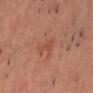{
  "biopsy_status": "not biopsied; imaged during a skin examination",
  "lesion_size": {
    "long_diameter_mm_approx": 3.0
  },
  "image": {
    "source": "total-body photography crop",
    "field_of_view_mm": 15
  },
  "automated_metrics": {
    "area_mm2_approx": 3.0,
    "shape_asymmetry": 0.35,
    "nevus_likeness_0_100": 0,
    "lesion_detection_confidence_0_100": 100
  },
  "lighting": "cross-polarized",
  "site": "chest",
  "patient": {
    "sex": "male",
    "age_approx": 50
  }
}